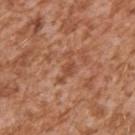Recorded during total-body skin imaging; not selected for excision or biopsy.
Approximately 4 mm at its widest.
The lesion is located on the right upper arm.
Imaged with white-light lighting.
A male subject, aged around 45.
Cropped from a total-body skin-imaging series; the visible field is about 15 mm.